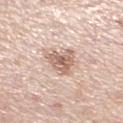  biopsy_status: not biopsied; imaged during a skin examination
  patient:
    sex: female
    age_approx: 65
  image:
    source: total-body photography crop
    field_of_view_mm: 15
  site: left lower leg